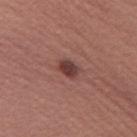Assessment:
The lesion was tiled from a total-body skin photograph and was not biopsied.
Acquisition and patient details:
The lesion's longest dimension is about 2.5 mm. On the left thigh. A lesion tile, about 15 mm wide, cut from a 3D total-body photograph. A female subject aged around 60. The tile uses white-light illumination.